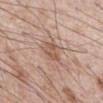* follow-up: no biopsy performed (imaged during a skin exam)
* imaging modality: ~15 mm crop, total-body skin-cancer survey
* automated metrics: an average lesion color of about L≈56 a*≈19 b*≈29 (CIELAB), roughly 8 lightness units darker than nearby skin, and a normalized lesion–skin contrast near 6
* anatomic site: the abdomen
* patient: male, roughly 70 years of age